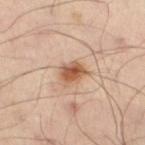  biopsy_status: not biopsied; imaged during a skin examination
  image:
    source: total-body photography crop
    field_of_view_mm: 15
  lesion_size:
    long_diameter_mm_approx: 3.0
  automated_metrics:
    cielab_L: 55
    cielab_a: 21
    cielab_b: 33
    vs_skin_darker_L: 13.0
    vs_skin_contrast_norm: 9.5
    peripheral_color_asymmetry: 1.5
    nevus_likeness_0_100: 95
  site: lower back
  patient:
    sex: male
    age_approx: 50